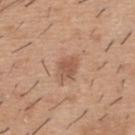{"biopsy_status": "not biopsied; imaged during a skin examination", "patient": {"sex": "male", "age_approx": 40}, "image": {"source": "total-body photography crop", "field_of_view_mm": 15}, "site": "upper back"}